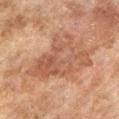Acquisition and patient details:
The tile uses cross-polarized illumination. The lesion is on the left lower leg. A 15 mm crop from a total-body photograph taken for skin-cancer surveillance. Approximately 8 mm at its widest. A female subject, aged 58 to 62.